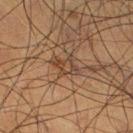Captured during whole-body skin photography for melanoma surveillance; the lesion was not biopsied.
The tile uses cross-polarized illumination.
The recorded lesion diameter is about 3 mm.
Located on the right thigh.
Automated tile analysis of the lesion measured an average lesion color of about L≈33 a*≈14 b*≈24 (CIELAB), about 6 CIELAB-L* units darker than the surrounding skin, and a normalized border contrast of about 6. The software also gave a border-irregularity rating of about 4/10, a color-variation rating of about 3/10, and a peripheral color-asymmetry measure near 0.5. The analysis additionally found a classifier nevus-likeness of about 0/100 and a detector confidence of about 50 out of 100 that the crop contains a lesion.
A 15 mm close-up extracted from a 3D total-body photography capture.
The subject is a male about 50 years old.